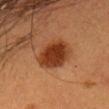This lesion was catalogued during total-body skin photography and was not selected for biopsy. The total-body-photography lesion software estimated a mean CIELAB color near L≈32 a*≈23 b*≈31, a lesion–skin lightness drop of about 12, and a normalized lesion–skin contrast near 11. It also reported a nevus-likeness score of about 100/100 and lesion-presence confidence of about 100/100. A female subject, approximately 55 years of age. A 15 mm close-up tile from a total-body photography series done for melanoma screening. On the head or neck.